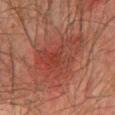No biopsy was performed on this lesion — it was imaged during a full skin examination and was not determined to be concerning.
About 7 mm across.
Cropped from a total-body skin-imaging series; the visible field is about 15 mm.
A male subject, roughly 75 years of age.
The lesion-visualizer software estimated a mean CIELAB color near L≈34 a*≈25 b*≈25, a lesion–skin lightness drop of about 6, and a normalized lesion–skin contrast near 5.5. It also reported an automated nevus-likeness rating near 5 out of 100 and a lesion-detection confidence of about 100/100.
Captured under cross-polarized illumination.
The lesion is on the chest.